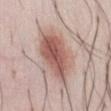image:
  source: total-body photography crop
  field_of_view_mm: 15
patient:
  sex: male
  age_approx: 25
automated_metrics:
  cielab_L: 57
  cielab_a: 21
  cielab_b: 24
  vs_skin_darker_L: 13.0
  vs_skin_contrast_norm: 9.0
  border_irregularity_0_10: 2.5
  color_variation_0_10: 6.0
  peripheral_color_asymmetry: 2.0
lighting: white-light
site: abdomen